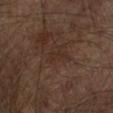Case summary:
• biopsy status: catalogued during a skin exam; not biopsied
• body site: the right forearm
• image source: ~15 mm crop, total-body skin-cancer survey
• subject: male, about 65 years old